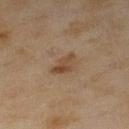notes=catalogued during a skin exam; not biopsied
patient=female, aged around 60
location=the leg
acquisition=15 mm crop, total-body photography
tile lighting=cross-polarized